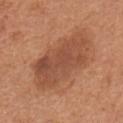Q: Is there a histopathology result?
A: catalogued during a skin exam; not biopsied
Q: What are the patient's age and sex?
A: male, aged 63 to 67
Q: Where on the body is the lesion?
A: the mid back
Q: How was this image acquired?
A: 15 mm crop, total-body photography
Q: How large is the lesion?
A: about 8.5 mm